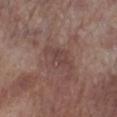Notes:
- tile lighting: white-light
- acquisition: total-body-photography crop, ~15 mm field of view
- subject: male, roughly 70 years of age
- anatomic site: the right lower leg
- size: about 4.5 mm